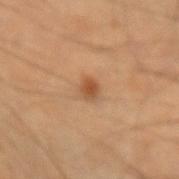A roughly 15 mm field-of-view crop from a total-body skin photograph.
About 2 mm across.
On the left forearm.
The tile uses cross-polarized illumination.
A male subject aged approximately 60.